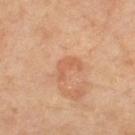Captured during whole-body skin photography for melanoma surveillance; the lesion was not biopsied.
Located on the left thigh.
Approximately 3.5 mm at its widest.
A lesion tile, about 15 mm wide, cut from a 3D total-body photograph.
This is a cross-polarized tile.
The patient is a female about 65 years old.